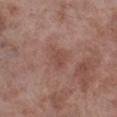Clinical impression:
Captured during whole-body skin photography for melanoma surveillance; the lesion was not biopsied.
Image and clinical context:
The total-body-photography lesion software estimated a lesion area of about 6 mm², a shape eccentricity near 0.6, and a symmetry-axis asymmetry near 0.25. The software also gave internal color variation of about 2.5 on a 0–10 scale and peripheral color asymmetry of about 1. This is a white-light tile. Measured at roughly 3 mm in maximum diameter. A 15 mm crop from a total-body photograph taken for skin-cancer surveillance. The patient is a male aged 53–57. From the right lower leg.